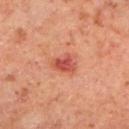<case>
  <biopsy_status>not biopsied; imaged during a skin examination</biopsy_status>
  <image>
    <source>total-body photography crop</source>
    <field_of_view_mm>15</field_of_view_mm>
  </image>
  <site>left lower leg</site>
  <patient>
    <sex>male</sex>
    <age_approx>60</age_approx>
  </patient>
</case>